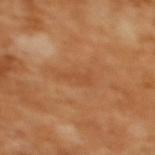Recorded during total-body skin imaging; not selected for excision or biopsy. The subject is a female aged approximately 55. Captured under cross-polarized illumination. On the upper back. Cropped from a total-body skin-imaging series; the visible field is about 15 mm.